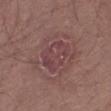biopsy_status: not biopsied; imaged during a skin examination
patient:
  sex: male
  age_approx: 55
image:
  source: total-body photography crop
  field_of_view_mm: 15
lighting: white-light
site: right thigh